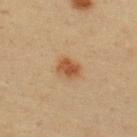The lesion was photographed on a routine skin check and not biopsied; there is no pathology result. A region of skin cropped from a whole-body photographic capture, roughly 15 mm wide. Measured at roughly 3 mm in maximum diameter. A male patient, aged around 40. Located on the upper back. The total-body-photography lesion software estimated an average lesion color of about L≈47 a*≈21 b*≈34 (CIELAB) and about 10 CIELAB-L* units darker than the surrounding skin. It also reported a border-irregularity index near 1.5/10 and a peripheral color-asymmetry measure near 1. This is a cross-polarized tile.